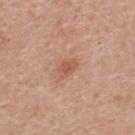Background: Approximately 2.5 mm at its widest. The tile uses white-light illumination. A 15 mm crop from a total-body photograph taken for skin-cancer surveillance. Located on the upper back. The total-body-photography lesion software estimated an outline eccentricity of about 0.75 (0 = round, 1 = elongated) and a shape-asymmetry score of about 0.3 (0 = symmetric). A male patient, roughly 55 years of age.